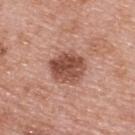Imaged during a routine full-body skin examination; the lesion was not biopsied and no histopathology is available. The subject is a female aged 58–62. A close-up tile cropped from a whole-body skin photograph, about 15 mm across. Imaged with white-light lighting. About 4 mm across. The lesion-visualizer software estimated a mean CIELAB color near L≈50 a*≈24 b*≈28, roughly 14 lightness units darker than nearby skin, and a normalized lesion–skin contrast near 9.5. It also reported a color-variation rating of about 5/10 and a peripheral color-asymmetry measure near 2. From the upper back.